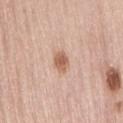| field | value |
|---|---|
| biopsy status | total-body-photography surveillance lesion; no biopsy |
| subject | female, aged 63 to 67 |
| imaging modality | 15 mm crop, total-body photography |
| size | about 3 mm |
| anatomic site | the lower back |
| automated lesion analysis | radial color variation of about 0.5 |